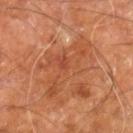  biopsy_status: not biopsied; imaged during a skin examination
  image:
    source: total-body photography crop
    field_of_view_mm: 15
  site: right leg
  patient:
    sex: male
    age_approx: 60
  lesion_size:
    long_diameter_mm_approx: 8.0
  lighting: cross-polarized
  automated_metrics:
    border_irregularity_0_10: 7.5
    color_variation_0_10: 5.5
    nevus_likeness_0_100: 0
    lesion_detection_confidence_0_100: 100